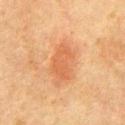biopsy status = catalogued during a skin exam; not biopsied
acquisition = ~15 mm tile from a whole-body skin photo
location = the chest
diameter = about 4.5 mm
patient = male, aged 78–82